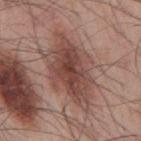{"biopsy_status": "not biopsied; imaged during a skin examination", "site": "mid back", "image": {"source": "total-body photography crop", "field_of_view_mm": 15}, "patient": {"sex": "male", "age_approx": 55}}